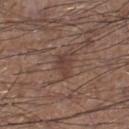Cropped from a whole-body photographic skin survey; the tile spans about 15 mm. A male subject, about 60 years old. From the right lower leg.